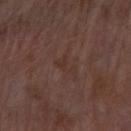Q: Patient demographics?
A: male, about 70 years old
Q: Where on the body is the lesion?
A: the left forearm
Q: Illumination type?
A: white-light
Q: How large is the lesion?
A: about 2.5 mm
Q: What kind of image is this?
A: total-body-photography crop, ~15 mm field of view